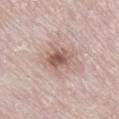Clinical impression:
Recorded during total-body skin imaging; not selected for excision or biopsy.
Context:
A male patient aged approximately 80. On the right lower leg. Captured under white-light illumination. Measured at roughly 3 mm in maximum diameter. A close-up tile cropped from a whole-body skin photograph, about 15 mm across.